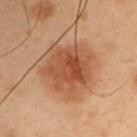<lesion>
  <biopsy_status>not biopsied; imaged during a skin examination</biopsy_status>
  <lighting>cross-polarized</lighting>
  <patient>
    <sex>male</sex>
    <age_approx>55</age_approx>
  </patient>
  <image>
    <source>total-body photography crop</source>
    <field_of_view_mm>15</field_of_view_mm>
  </image>
  <lesion_size>
    <long_diameter_mm_approx>5.5</long_diameter_mm_approx>
  </lesion_size>
  <site>arm</site>
</lesion>